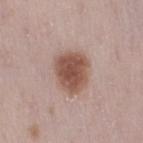Case summary:
– notes: imaged on a skin check; not biopsied
– illumination: white-light
– lesion size: ~5 mm (longest diameter)
– subject: female, aged 28 to 32
– location: the leg
– image: total-body-photography crop, ~15 mm field of view
– image-analysis metrics: an area of roughly 15 mm², a shape eccentricity near 0.6, and two-axis asymmetry of about 0.15; a nevus-likeness score of about 100/100